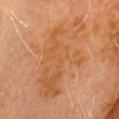Impression: Imaged during a routine full-body skin examination; the lesion was not biopsied and no histopathology is available. Image and clinical context: This is a cross-polarized tile. A male patient, aged 68–72. From the head or neck. Cropped from a total-body skin-imaging series; the visible field is about 15 mm.